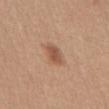biopsy_status: not biopsied; imaged during a skin examination
site: front of the torso
automated_metrics:
  eccentricity: 0.75
  shape_asymmetry: 0.2
  cielab_L: 53
  cielab_a: 22
  cielab_b: 31
  vs_skin_darker_L: 10.0
  vs_skin_contrast_norm: 7.5
  border_irregularity_0_10: 2.0
  peripheral_color_asymmetry: 0.5
  nevus_likeness_0_100: 90
  lesion_detection_confidence_0_100: 100
lesion_size:
  long_diameter_mm_approx: 2.5
image:
  source: total-body photography crop
  field_of_view_mm: 15
patient:
  sex: female
  age_approx: 35